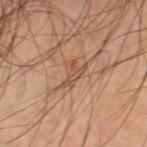Imaged during a routine full-body skin examination; the lesion was not biopsied and no histopathology is available.
Cropped from a whole-body photographic skin survey; the tile spans about 15 mm.
Imaged with cross-polarized lighting.
Longest diameter approximately 3.5 mm.
The lesion is on the right lower leg.
The subject is a male aged around 50.